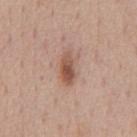Part of a total-body skin-imaging series; this lesion was reviewed on a skin check and was not flagged for biopsy.
The lesion's longest dimension is about 4.5 mm.
A close-up tile cropped from a whole-body skin photograph, about 15 mm across.
From the front of the torso.
The patient is a male in their 60s.